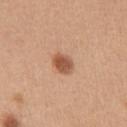Impression: Part of a total-body skin-imaging series; this lesion was reviewed on a skin check and was not flagged for biopsy. Image and clinical context: The total-body-photography lesion software estimated an area of roughly 5 mm², a shape eccentricity near 0.65, and a symmetry-axis asymmetry near 0.2. The software also gave a nevus-likeness score of about 100/100 and a lesion-detection confidence of about 100/100. The subject is a female aged 28–32. This image is a 15 mm lesion crop taken from a total-body photograph. The tile uses white-light illumination. The lesion is on the chest.